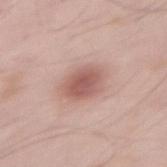The lesion-visualizer software estimated a classifier nevus-likeness of about 90/100 and a lesion-detection confidence of about 100/100.
Imaged with white-light lighting.
The lesion is located on the abdomen.
About 4 mm across.
The patient is a male in their mid- to late 50s.
A lesion tile, about 15 mm wide, cut from a 3D total-body photograph.